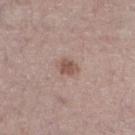| field | value |
|---|---|
| workup | total-body-photography surveillance lesion; no biopsy |
| image | 15 mm crop, total-body photography |
| tile lighting | white-light illumination |
| location | the leg |
| patient | male, in their mid-50s |